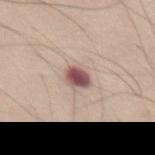biopsy status: imaged on a skin check; not biopsied | body site: the abdomen | subject: male, about 65 years old | image source: 15 mm crop, total-body photography | lesion size: ≈2.5 mm.